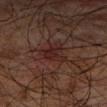Clinical impression:
Part of a total-body skin-imaging series; this lesion was reviewed on a skin check and was not flagged for biopsy.
Context:
A male subject aged 73–77. This image is a 15 mm lesion crop taken from a total-body photograph. Captured under cross-polarized illumination. The lesion's longest dimension is about 4 mm. Located on the arm.